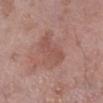* biopsy status — imaged on a skin check; not biopsied
* image-analysis metrics — a lesion area of about 6 mm², an outline eccentricity of about 0.95 (0 = round, 1 = elongated), and a symmetry-axis asymmetry near 0.4; border irregularity of about 5.5 on a 0–10 scale, a color-variation rating of about 1/10, and a peripheral color-asymmetry measure near 0.5
* lighting — white-light illumination
* lesion size — about 4.5 mm
* anatomic site — the right lower leg
* subject — male, aged 68 to 72
* acquisition — 15 mm crop, total-body photography